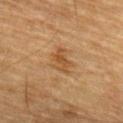Case summary:
• notes: total-body-photography surveillance lesion; no biopsy
• lesion size: ~3 mm (longest diameter)
• patient: male, approximately 85 years of age or older
• image: total-body-photography crop, ~15 mm field of view
• tile lighting: cross-polarized illumination
• body site: the arm